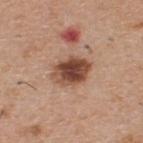Clinical impression:
Captured during whole-body skin photography for melanoma surveillance; the lesion was not biopsied.
Background:
Automated tile analysis of the lesion measured a mean CIELAB color near L≈47 a*≈22 b*≈29. And it measured internal color variation of about 6.5 on a 0–10 scale and radial color variation of about 2. The analysis additionally found a nevus-likeness score of about 85/100. From the upper back. A male patient aged around 60. Captured under white-light illumination. A roughly 15 mm field-of-view crop from a total-body skin photograph. About 4.5 mm across.